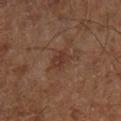No biopsy was performed on this lesion — it was imaged during a full skin examination and was not determined to be concerning.
A roughly 15 mm field-of-view crop from a total-body skin photograph.
The total-body-photography lesion software estimated a border-irregularity index near 2.5/10 and a peripheral color-asymmetry measure near 0.5. It also reported a nevus-likeness score of about 5/100 and a detector confidence of about 100 out of 100 that the crop contains a lesion.
The tile uses cross-polarized illumination.
About 2.5 mm across.
The patient is roughly 65 years of age.
Located on the left lower leg.